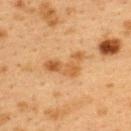{"lighting": "cross-polarized", "site": "upper back", "image": {"source": "total-body photography crop", "field_of_view_mm": 15}, "lesion_size": {"long_diameter_mm_approx": 5.0}, "patient": {"sex": "female", "age_approx": 40}}